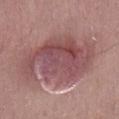Background:
On the mid back. A male patient, in their mid-50s. Imaged with white-light lighting. A region of skin cropped from a whole-body photographic capture, roughly 15 mm wide.